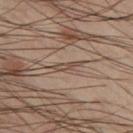notes: no biopsy performed (imaged during a skin exam)
image: ~15 mm tile from a whole-body skin photo
TBP lesion metrics: an area of roughly 2 mm², an outline eccentricity of about 0.95 (0 = round, 1 = elongated), and two-axis asymmetry of about 0.35; an average lesion color of about L≈48 a*≈14 b*≈25 (CIELAB), roughly 9 lightness units darker than nearby skin, and a normalized border contrast of about 7; border irregularity of about 5 on a 0–10 scale
location: the left thigh
lesion size: ≈3 mm
patient: male, aged 38–42